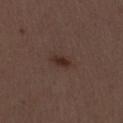<lesion>
  <biopsy_status>not biopsied; imaged during a skin examination</biopsy_status>
  <lighting>white-light</lighting>
  <image>
    <source>total-body photography crop</source>
    <field_of_view_mm>15</field_of_view_mm>
  </image>
  <site>abdomen</site>
  <automated_metrics>
    <border_irregularity_0_10>2.0</border_irregularity_0_10>
    <color_variation_0_10>2.0</color_variation_0_10>
    <peripheral_color_asymmetry>0.5</peripheral_color_asymmetry>
    <nevus_likeness_0_100>90</nevus_likeness_0_100>
    <lesion_detection_confidence_0_100>100</lesion_detection_confidence_0_100>
  </automated_metrics>
  <lesion_size>
    <long_diameter_mm_approx>3.0</long_diameter_mm_approx>
  </lesion_size>
  <patient>
    <sex>female</sex>
    <age_approx>50</age_approx>
  </patient>
</lesion>